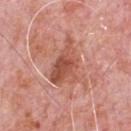Assessment: This lesion was catalogued during total-body skin photography and was not selected for biopsy. Background: The total-body-photography lesion software estimated an area of roughly 12 mm², an eccentricity of roughly 0.85, and a shape-asymmetry score of about 0.35 (0 = symmetric). The software also gave a lesion color around L≈53 a*≈27 b*≈30 in CIELAB, roughly 11 lightness units darker than nearby skin, and a normalized lesion–skin contrast near 7.5. And it measured an automated nevus-likeness rating near 20 out of 100 and a detector confidence of about 100 out of 100 that the crop contains a lesion. Cropped from a total-body skin-imaging series; the visible field is about 15 mm. The lesion is located on the front of the torso. Imaged with white-light lighting. Longest diameter approximately 5.5 mm. A male subject roughly 80 years of age.